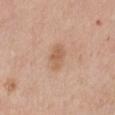- notes: total-body-photography surveillance lesion; no biopsy
- lighting: white-light illumination
- subject: male, approximately 60 years of age
- image: ~15 mm crop, total-body skin-cancer survey
- body site: the chest